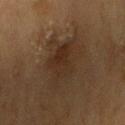Acquisition and patient details: A 15 mm crop from a total-body photograph taken for skin-cancer surveillance. This is a cross-polarized tile. The patient is a male aged 83 or older. The lesion-visualizer software estimated an automated nevus-likeness rating near 45 out of 100 and a detector confidence of about 95 out of 100 that the crop contains a lesion. About 7 mm across. The lesion is on the right upper arm.